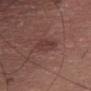  image:
    source: total-body photography crop
    field_of_view_mm: 15
  patient:
    sex: male
    age_approx: 75
  lighting: white-light
  site: right thigh
  lesion_size:
    long_diameter_mm_approx: 3.0